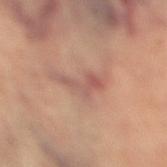Notes:
* biopsy status — no biopsy performed (imaged during a skin exam)
* subject — female, roughly 65 years of age
* imaging modality — 15 mm crop, total-body photography
* location — the leg